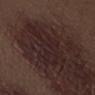Imaged during a routine full-body skin examination; the lesion was not biopsied and no histopathology is available.